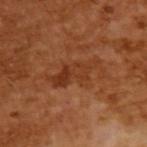Assessment:
This lesion was catalogued during total-body skin photography and was not selected for biopsy.
Acquisition and patient details:
A male subject aged 63–67. This image is a 15 mm lesion crop taken from a total-body photograph. Automated tile analysis of the lesion measured a border-irregularity index near 7/10, a color-variation rating of about 5/10, and a peripheral color-asymmetry measure near 1.5. The analysis additionally found an automated nevus-likeness rating near 0 out of 100. Captured under cross-polarized illumination. Approximately 6 mm at its widest.